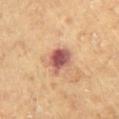Clinical impression:
Captured during whole-body skin photography for melanoma surveillance; the lesion was not biopsied.
Clinical summary:
This is a cross-polarized tile. Cropped from a total-body skin-imaging series; the visible field is about 15 mm. On the right arm. A female subject, roughly 80 years of age.